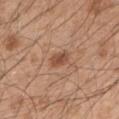patient=male, about 50 years old
site=the arm
diameter=≈3 mm
image source=~15 mm crop, total-body skin-cancer survey
tile lighting=white-light